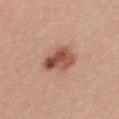Case summary:
• notes — imaged on a skin check; not biopsied
• patient — female, aged around 20
• image source — total-body-photography crop, ~15 mm field of view
• site — the upper back